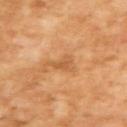notes: no biopsy performed (imaged during a skin exam); lighting: cross-polarized illumination; size: ~2.5 mm (longest diameter); imaging modality: 15 mm crop, total-body photography; subject: male, aged 63–67.